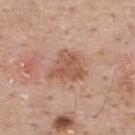Background:
Automated tile analysis of the lesion measured a mean CIELAB color near L≈55 a*≈23 b*≈30, about 10 CIELAB-L* units darker than the surrounding skin, and a normalized border contrast of about 7. The analysis additionally found a nevus-likeness score of about 15/100 and lesion-presence confidence of about 100/100. The tile uses white-light illumination. A 15 mm close-up extracted from a 3D total-body photography capture. A male patient, approximately 60 years of age. The lesion is on the upper back.